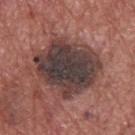workup: catalogued during a skin exam; not biopsied
illumination: white-light
diameter: about 7.5 mm
body site: the upper back
subject: male, roughly 75 years of age
automated metrics: a border-irregularity rating of about 2/10, internal color variation of about 7 on a 0–10 scale, and radial color variation of about 2; a classifier nevus-likeness of about 0/100 and a lesion-detection confidence of about 100/100
acquisition: ~15 mm tile from a whole-body skin photo